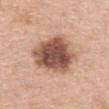notes=imaged on a skin check; not biopsied | location=the chest | imaging modality=~15 mm tile from a whole-body skin photo | size=about 5.5 mm | tile lighting=white-light illumination | patient=female, roughly 55 years of age.